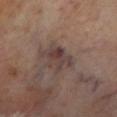Q: Was this lesion biopsied?
A: imaged on a skin check; not biopsied
Q: Who is the patient?
A: male, in their 70s
Q: Illumination type?
A: cross-polarized illumination
Q: Where on the body is the lesion?
A: the leg
Q: What did automated image analysis measure?
A: a border-irregularity rating of about 5/10, a color-variation rating of about 4.5/10, and a peripheral color-asymmetry measure near 1.5; a classifier nevus-likeness of about 0/100
Q: How large is the lesion?
A: ≈4 mm
Q: How was this image acquired?
A: ~15 mm tile from a whole-body skin photo